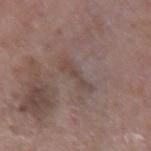Recorded during total-body skin imaging; not selected for excision or biopsy. The subject is a male aged 58–62. Automated tile analysis of the lesion measured border irregularity of about 6 on a 0–10 scale, a within-lesion color-variation index near 0.5/10, and radial color variation of about 0. It also reported lesion-presence confidence of about 85/100. A lesion tile, about 15 mm wide, cut from a 3D total-body photograph. Imaged with white-light lighting. On the right forearm.